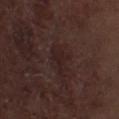No biopsy was performed on this lesion — it was imaged during a full skin examination and was not determined to be concerning. Imaged with white-light lighting. Automated tile analysis of the lesion measured an area of roughly 4.5 mm², an outline eccentricity of about 0.85 (0 = round, 1 = elongated), and a symmetry-axis asymmetry near 0.55. The analysis additionally found a lesion color around L≈21 a*≈16 b*≈15 in CIELAB, roughly 5 lightness units darker than nearby skin, and a lesion-to-skin contrast of about 6 (normalized; higher = more distinct). It also reported border irregularity of about 5.5 on a 0–10 scale and a color-variation rating of about 1/10. A male patient, aged approximately 70. From the right thigh. A roughly 15 mm field-of-view crop from a total-body skin photograph.